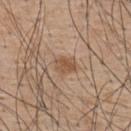Impression: The lesion was tiled from a total-body skin photograph and was not biopsied. Clinical summary: A male subject in their mid-50s. A region of skin cropped from a whole-body photographic capture, roughly 15 mm wide. Measured at roughly 3 mm in maximum diameter. On the back.